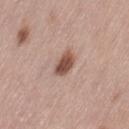Assessment:
The lesion was tiled from a total-body skin photograph and was not biopsied.
Clinical summary:
The recorded lesion diameter is about 3 mm. Imaged with white-light lighting. A female patient about 65 years old. The lesion is on the leg. A region of skin cropped from a whole-body photographic capture, roughly 15 mm wide.